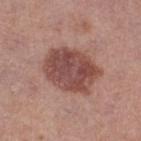<lesion>
  <biopsy_status>not biopsied; imaged during a skin examination</biopsy_status>
  <patient>
    <sex>female</sex>
    <age_approx>40</age_approx>
  </patient>
  <site>right thigh</site>
  <image>
    <source>total-body photography crop</source>
    <field_of_view_mm>15</field_of_view_mm>
  </image>
</lesion>